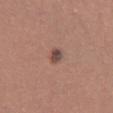Q: Was a biopsy performed?
A: no biopsy performed (imaged during a skin exam)
Q: Automated lesion metrics?
A: an area of roughly 3 mm², a shape eccentricity near 0.85, and a symmetry-axis asymmetry near 0.2; an average lesion color of about L≈46 a*≈19 b*≈23 (CIELAB) and roughly 12 lightness units darker than nearby skin; border irregularity of about 2 on a 0–10 scale, internal color variation of about 3.5 on a 0–10 scale, and radial color variation of about 1; a nevus-likeness score of about 70/100 and a detector confidence of about 100 out of 100 that the crop contains a lesion
Q: Illumination type?
A: white-light illumination
Q: What is the anatomic site?
A: the right thigh
Q: What kind of image is this?
A: ~15 mm crop, total-body skin-cancer survey
Q: Lesion size?
A: ~2.5 mm (longest diameter)
Q: What are the patient's age and sex?
A: female, aged around 35